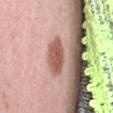Clinical impression: Imaged during a routine full-body skin examination; the lesion was not biopsied and no histopathology is available. Background: Captured under white-light illumination. A 15 mm crop from a total-body photograph taken for skin-cancer surveillance. A female patient approximately 40 years of age. The recorded lesion diameter is about 4.5 mm. The lesion is on the lower back. The lesion-visualizer software estimated a lesion area of about 9 mm², an eccentricity of roughly 0.8, and a symmetry-axis asymmetry near 0.1. And it measured a border-irregularity rating of about 1.5/10, a within-lesion color-variation index near 3.5/10, and radial color variation of about 1. The software also gave a classifier nevus-likeness of about 90/100 and a lesion-detection confidence of about 100/100.